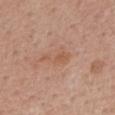The lesion was tiled from a total-body skin photograph and was not biopsied. A male subject aged 53–57. This image is a 15 mm lesion crop taken from a total-body photograph. On the back. Captured under white-light illumination.